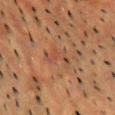Q: Was a biopsy performed?
A: catalogued during a skin exam; not biopsied
Q: Automated lesion metrics?
A: a lesion area of about 4.5 mm² and a symmetry-axis asymmetry near 0.5; roughly 5 lightness units darker than nearby skin; a border-irregularity rating of about 5.5/10 and radial color variation of about 0; a lesion-detection confidence of about 75/100
Q: What is the anatomic site?
A: the upper back
Q: What kind of image is this?
A: ~15 mm tile from a whole-body skin photo
Q: Who is the patient?
A: male, aged approximately 55
Q: What lighting was used for the tile?
A: cross-polarized illumination
Q: How large is the lesion?
A: ≈3.5 mm